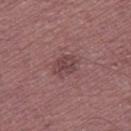Notes:
• image · 15 mm crop, total-body photography
• TBP lesion metrics · a mean CIELAB color near L≈42 a*≈21 b*≈17, about 8 CIELAB-L* units darker than the surrounding skin, and a normalized lesion–skin contrast near 6.5; border irregularity of about 2.5 on a 0–10 scale and radial color variation of about 1
• anatomic site · the right thigh
• lighting · white-light illumination
• subject · male, approximately 60 years of age
• lesion diameter · ~3 mm (longest diameter)